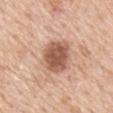Findings:
- biopsy status — catalogued during a skin exam; not biopsied
- illumination — white-light illumination
- automated metrics — a footprint of about 13 mm², an outline eccentricity of about 0.45 (0 = round, 1 = elongated), and a symmetry-axis asymmetry near 0.15; a border-irregularity index near 1.5/10, a color-variation rating of about 3.5/10, and peripheral color asymmetry of about 1
- body site — the mid back
- lesion size — ~4 mm (longest diameter)
- image — total-body-photography crop, ~15 mm field of view
- subject — male, about 60 years old